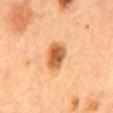Impression: Captured during whole-body skin photography for melanoma surveillance; the lesion was not biopsied. Context: A female patient aged approximately 60. The recorded lesion diameter is about 3.5 mm. A region of skin cropped from a whole-body photographic capture, roughly 15 mm wide. An algorithmic analysis of the crop reported a border-irregularity rating of about 2/10, a within-lesion color-variation index near 4.5/10, and a peripheral color-asymmetry measure near 1.5. Located on the abdomen.